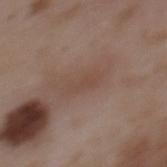Assessment:
The lesion was photographed on a routine skin check and not biopsied; there is no pathology result.
Clinical summary:
The total-body-photography lesion software estimated a lesion area of about 5.5 mm², a shape eccentricity near 0.9, and two-axis asymmetry of about 0.3. The analysis additionally found an average lesion color of about L≈46 a*≈17 b*≈24 (CIELAB), roughly 5 lightness units darker than nearby skin, and a lesion-to-skin contrast of about 4.5 (normalized; higher = more distinct). And it measured a nevus-likeness score of about 0/100 and a detector confidence of about 95 out of 100 that the crop contains a lesion. A roughly 15 mm field-of-view crop from a total-body skin photograph. The lesion is on the mid back. This is a white-light tile. About 4 mm across. A male subject aged 48–52.